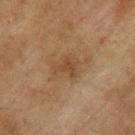Clinical impression:
Imaged during a routine full-body skin examination; the lesion was not biopsied and no histopathology is available.
Clinical summary:
A male subject aged 73 to 77. Measured at roughly 3 mm in maximum diameter. From the left upper arm. A region of skin cropped from a whole-body photographic capture, roughly 15 mm wide. The total-body-photography lesion software estimated a border-irregularity index near 6/10 and a peripheral color-asymmetry measure near 0.5. And it measured a detector confidence of about 100 out of 100 that the crop contains a lesion.